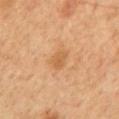No biopsy was performed on this lesion — it was imaged during a full skin examination and was not determined to be concerning.
The subject is a male in their 60s.
Automated image analysis of the tile measured a lesion color around L≈53 a*≈20 b*≈37 in CIELAB, roughly 7 lightness units darker than nearby skin, and a normalized border contrast of about 5.5. The analysis additionally found a classifier nevus-likeness of about 5/100 and a detector confidence of about 100 out of 100 that the crop contains a lesion.
The recorded lesion diameter is about 2.5 mm.
Captured under cross-polarized illumination.
Cropped from a total-body skin-imaging series; the visible field is about 15 mm.
The lesion is located on the chest.